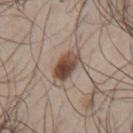Q: Was this lesion biopsied?
A: no biopsy performed (imaged during a skin exam)
Q: What kind of image is this?
A: total-body-photography crop, ~15 mm field of view
Q: Illumination type?
A: white-light
Q: Who is the patient?
A: male, aged around 50
Q: Lesion size?
A: about 4.5 mm
Q: Lesion location?
A: the mid back